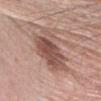Imaged during a routine full-body skin examination; the lesion was not biopsied and no histopathology is available.
This is a white-light tile.
Approximately 6 mm at its widest.
A roughly 15 mm field-of-view crop from a total-body skin photograph.
Located on the right forearm.
A male patient, approximately 75 years of age.